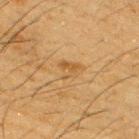| feature | finding |
|---|---|
| notes | total-body-photography surveillance lesion; no biopsy |
| image source | ~15 mm crop, total-body skin-cancer survey |
| lighting | cross-polarized illumination |
| location | the right upper arm |
| diameter | about 3 mm |
| subject | male, aged around 35 |